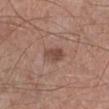Clinical impression: Captured during whole-body skin photography for melanoma surveillance; the lesion was not biopsied. Image and clinical context: A 15 mm crop from a total-body photograph taken for skin-cancer surveillance. Located on the left lower leg. A male subject, approximately 55 years of age.